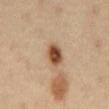notes — imaged on a skin check; not biopsied | body site — the abdomen | diameter — ≈3 mm | image source — ~15 mm tile from a whole-body skin photo | automated metrics — a mean CIELAB color near L≈49 a*≈21 b*≈33 and a normalized border contrast of about 12; a classifier nevus-likeness of about 100/100 and a detector confidence of about 100 out of 100 that the crop contains a lesion | patient — male, aged around 40 | tile lighting — cross-polarized.